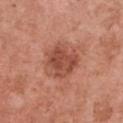Part of a total-body skin-imaging series; this lesion was reviewed on a skin check and was not flagged for biopsy. A female subject, aged around 50. Approximately 4 mm at its widest. Cropped from a total-body skin-imaging series; the visible field is about 15 mm. On the chest.